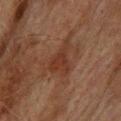Case summary:
* follow-up — catalogued during a skin exam; not biopsied
* body site — the back
* patient — male, in their mid- to late 70s
* lighting — cross-polarized
* imaging modality — total-body-photography crop, ~15 mm field of view
* diameter — ≈4.5 mm